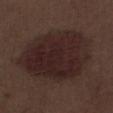follow-up = imaged on a skin check; not biopsied | automated metrics = lesion-presence confidence of about 100/100 | lighting = white-light | image source = total-body-photography crop, ~15 mm field of view | anatomic site = the left thigh | subject = male, aged 68–72 | size = ≈9 mm.